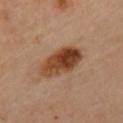Assessment: Recorded during total-body skin imaging; not selected for excision or biopsy. Clinical summary: This image is a 15 mm lesion crop taken from a total-body photograph. A female patient, roughly 60 years of age. The lesion is located on the chest.